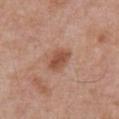{"biopsy_status": "not biopsied; imaged during a skin examination", "lighting": "white-light", "image": {"source": "total-body photography crop", "field_of_view_mm": 15}, "automated_metrics": {"area_mm2_approx": 5.5, "eccentricity": 0.7, "shape_asymmetry": 0.25}, "lesion_size": {"long_diameter_mm_approx": 3.0}, "site": "front of the torso", "patient": {"sex": "male", "age_approx": 70}}